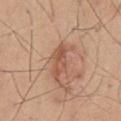Clinical impression:
This lesion was catalogued during total-body skin photography and was not selected for biopsy.
Image and clinical context:
The patient is a male in their mid- to late 40s. On the mid back. A lesion tile, about 15 mm wide, cut from a 3D total-body photograph. Automated tile analysis of the lesion measured two-axis asymmetry of about 0.35. It also reported a border-irregularity rating of about 4.5/10. It also reported an automated nevus-likeness rating near 10 out of 100. The tile uses white-light illumination.